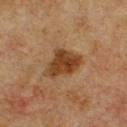• workup — imaged on a skin check; not biopsied